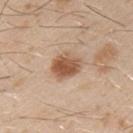follow-up — imaged on a skin check; not biopsied
subject — male, approximately 30 years of age
image source — total-body-photography crop, ~15 mm field of view
diameter — ~4 mm (longest diameter)
body site — the left upper arm